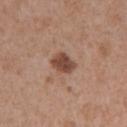Clinical summary:
About 2.5 mm across. The lesion is on the left upper arm. A female subject, aged approximately 40. Captured under white-light illumination. A 15 mm close-up extracted from a 3D total-body photography capture.